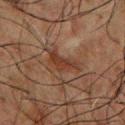{"biopsy_status": "not biopsied; imaged during a skin examination", "patient": {"sex": "male", "age_approx": 60}, "lesion_size": {"long_diameter_mm_approx": 4.5}, "image": {"source": "total-body photography crop", "field_of_view_mm": 15}, "site": "chest"}